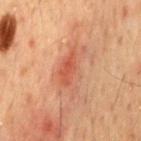biopsy_status: not biopsied; imaged during a skin examination
automated_metrics:
  cielab_L: 43
  cielab_a: 22
  cielab_b: 27
  vs_skin_darker_L: 7.0
  vs_skin_contrast_norm: 6.0
  border_irregularity_0_10: 5.5
  color_variation_0_10: 4.0
  peripheral_color_asymmetry: 1.0
  nevus_likeness_0_100: 5
  lesion_detection_confidence_0_100: 100
image:
  source: total-body photography crop
  field_of_view_mm: 15
site: chest
lesion_size:
  long_diameter_mm_approx: 5.5
patient:
  sex: male
  age_approx: 50